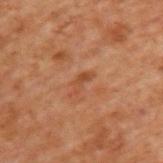Q: Was this lesion biopsied?
A: imaged on a skin check; not biopsied
Q: What is the lesion's diameter?
A: about 3 mm
Q: Patient demographics?
A: male, aged 68 to 72
Q: What is the anatomic site?
A: the upper back
Q: How was the tile lit?
A: cross-polarized illumination
Q: Automated lesion metrics?
A: a shape eccentricity near 0.9 and two-axis asymmetry of about 0.5; a nevus-likeness score of about 0/100
Q: How was this image acquired?
A: 15 mm crop, total-body photography